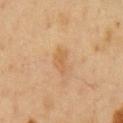biopsy_status: not biopsied; imaged during a skin examination
site: front of the torso
image:
  source: total-body photography crop
  field_of_view_mm: 15
patient:
  sex: male
  age_approx: 50
lesion_size:
  long_diameter_mm_approx: 3.5